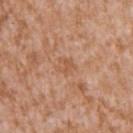workup = no biopsy performed (imaged during a skin exam); anatomic site = the left upper arm; subject = male, in their mid-40s; imaging modality = 15 mm crop, total-body photography.